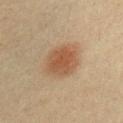A 15 mm close-up extracted from a 3D total-body photography capture.
The patient is a male aged approximately 40.
The lesion is on the arm.